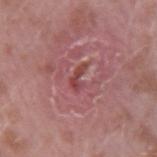biopsy_status: not biopsied; imaged during a skin examination